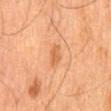Image and clinical context:
A 15 mm close-up extracted from a 3D total-body photography capture. A male subject, aged 63 to 67. The lesion is located on the back. Longest diameter approximately 3 mm. This is a cross-polarized tile.